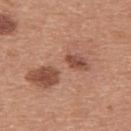Recorded during total-body skin imaging; not selected for excision or biopsy.
A male subject in their 30s.
The lesion is located on the upper back.
A 15 mm close-up tile from a total-body photography series done for melanoma screening.
Longest diameter approximately 9.5 mm.
The lesion-visualizer software estimated an average lesion color of about L≈54 a*≈22 b*≈29 (CIELAB), roughly 8 lightness units darker than nearby skin, and a normalized border contrast of about 6. The software also gave border irregularity of about 6 on a 0–10 scale, internal color variation of about 9 on a 0–10 scale, and radial color variation of about 3.5.
The tile uses white-light illumination.